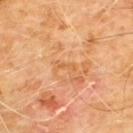| key | value |
|---|---|
| follow-up | imaged on a skin check; not biopsied |
| patient | male, aged 58–62 |
| site | the chest |
| image source | ~15 mm tile from a whole-body skin photo |